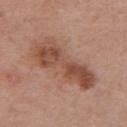This lesion was catalogued during total-body skin photography and was not selected for biopsy. A close-up tile cropped from a whole-body skin photograph, about 15 mm across. A male patient, in their 70s. About 7.5 mm across. The lesion is on the abdomen. The total-body-photography lesion software estimated a lesion area of about 20 mm², a shape eccentricity near 0.9, and a symmetry-axis asymmetry near 0.3. The analysis additionally found a lesion color around L≈49 a*≈22 b*≈29 in CIELAB, roughly 11 lightness units darker than nearby skin, and a lesion-to-skin contrast of about 8 (normalized; higher = more distinct). The analysis additionally found border irregularity of about 5 on a 0–10 scale, a within-lesion color-variation index near 5/10, and peripheral color asymmetry of about 1.5. The analysis additionally found a classifier nevus-likeness of about 5/100 and lesion-presence confidence of about 100/100. Captured under white-light illumination.